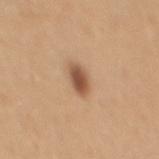Imaged during a routine full-body skin examination; the lesion was not biopsied and no histopathology is available. About 3 mm across. A 15 mm close-up extracted from a 3D total-body photography capture. Imaged with white-light lighting. The patient is a male about 50 years old. The lesion is on the mid back. Automated tile analysis of the lesion measured a lesion area of about 5 mm², a shape eccentricity near 0.8, and a symmetry-axis asymmetry near 0.2. The analysis additionally found a lesion color around L≈53 a*≈21 b*≈32 in CIELAB, roughly 14 lightness units darker than nearby skin, and a normalized border contrast of about 9.5. It also reported a border-irregularity rating of about 1.5/10, internal color variation of about 3.5 on a 0–10 scale, and a peripheral color-asymmetry measure near 1. The software also gave a classifier nevus-likeness of about 100/100.